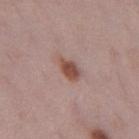Part of a total-body skin-imaging series; this lesion was reviewed on a skin check and was not flagged for biopsy.
The lesion is on the left thigh.
The patient is a female aged approximately 30.
A 15 mm crop from a total-body photograph taken for skin-cancer surveillance.
The tile uses white-light illumination.
The total-body-photography lesion software estimated a normalized border contrast of about 9. And it measured a color-variation rating of about 3/10 and a peripheral color-asymmetry measure near 1. The analysis additionally found lesion-presence confidence of about 100/100.